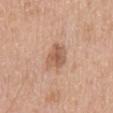follow-up: imaged on a skin check; not biopsied | diameter: about 3.5 mm | tile lighting: white-light illumination | anatomic site: the chest | image: total-body-photography crop, ~15 mm field of view | patient: male, aged around 65.